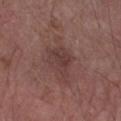Recorded during total-body skin imaging; not selected for excision or biopsy. The patient is a male aged 63 to 67. The lesion is located on the left forearm. Cropped from a whole-body photographic skin survey; the tile spans about 15 mm.